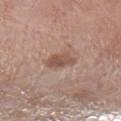Clinical impression:
Imaged during a routine full-body skin examination; the lesion was not biopsied and no histopathology is available.
Image and clinical context:
The lesion is located on the leg. A male subject aged 78 to 82. Cropped from a whole-body photographic skin survey; the tile spans about 15 mm.